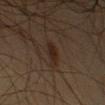Impression: No biopsy was performed on this lesion — it was imaged during a full skin examination and was not determined to be concerning. Image and clinical context: Approximately 2.5 mm at its widest. The total-body-photography lesion software estimated a lesion area of about 3.5 mm², an eccentricity of roughly 0.75, and a shape-asymmetry score of about 0.2 (0 = symmetric). The software also gave an automated nevus-likeness rating near 65 out of 100 and a detector confidence of about 100 out of 100 that the crop contains a lesion. A roughly 15 mm field-of-view crop from a total-body skin photograph. A male patient, in their mid- to late 30s. Captured under cross-polarized illumination. From the left upper arm.